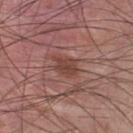Impression: Imaged during a routine full-body skin examination; the lesion was not biopsied and no histopathology is available. Context: The tile uses white-light illumination. The lesion is on the chest. A male subject approximately 25 years of age. The lesion's longest dimension is about 3.5 mm. Automated image analysis of the tile measured an outline eccentricity of about 0.7 (0 = round, 1 = elongated) and two-axis asymmetry of about 0.25. The analysis additionally found a color-variation rating of about 4.5/10 and peripheral color asymmetry of about 1.5. A 15 mm close-up extracted from a 3D total-body photography capture.